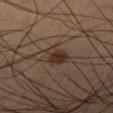Q: Was a biopsy performed?
A: catalogued during a skin exam; not biopsied
Q: What kind of image is this?
A: ~15 mm tile from a whole-body skin photo
Q: What is the lesion's diameter?
A: about 4 mm
Q: Where on the body is the lesion?
A: the right lower leg
Q: What lighting was used for the tile?
A: cross-polarized
Q: What did automated image analysis measure?
A: an eccentricity of roughly 0.8 and a shape-asymmetry score of about 0.35 (0 = symmetric); about 9 CIELAB-L* units darker than the surrounding skin and a normalized border contrast of about 9.5; an automated nevus-likeness rating near 90 out of 100 and lesion-presence confidence of about 100/100
Q: Who is the patient?
A: male, in their mid-60s